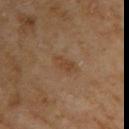follow-up: imaged on a skin check; not biopsied
lesion size: about 3.5 mm
site: the right upper arm
TBP lesion metrics: a shape eccentricity near 0.9 and two-axis asymmetry of about 0.3; a lesion color around L≈43 a*≈17 b*≈32 in CIELAB, about 6 CIELAB-L* units darker than the surrounding skin, and a lesion-to-skin contrast of about 5.5 (normalized; higher = more distinct); an automated nevus-likeness rating near 0 out of 100
acquisition: 15 mm crop, total-body photography
illumination: cross-polarized illumination
patient: female, aged around 60